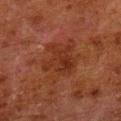notes: imaged on a skin check; not biopsied
site: the left lower leg
image source: ~15 mm tile from a whole-body skin photo
size: ≈4.5 mm
automated metrics: a lesion area of about 11 mm², an eccentricity of roughly 0.55, and a shape-asymmetry score of about 0.45 (0 = symmetric)
tile lighting: cross-polarized illumination
subject: male, aged 78–82